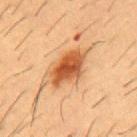The lesion was photographed on a routine skin check and not biopsied; there is no pathology result. A male patient about 55 years old. Cropped from a whole-body photographic skin survey; the tile spans about 15 mm. The lesion is located on the front of the torso. An algorithmic analysis of the crop reported a border-irregularity rating of about 2.5/10, a color-variation rating of about 5/10, and a peripheral color-asymmetry measure near 1.5. The lesion's longest dimension is about 5.5 mm. The tile uses cross-polarized illumination.